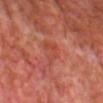Part of a total-body skin-imaging series; this lesion was reviewed on a skin check and was not flagged for biopsy. From the chest. A male subject, aged 58 to 62. Automated tile analysis of the lesion measured border irregularity of about 5.5 on a 0–10 scale and a within-lesion color-variation index near 2/10. It also reported a nevus-likeness score of about 0/100 and a lesion-detection confidence of about 95/100. A close-up tile cropped from a whole-body skin photograph, about 15 mm across.